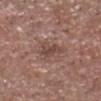workup = no biopsy performed (imaged during a skin exam)
diameter = ≈3.5 mm
automated lesion analysis = a border-irregularity rating of about 4.5/10, a within-lesion color-variation index near 2/10, and a peripheral color-asymmetry measure near 0.5; a detector confidence of about 100 out of 100 that the crop contains a lesion
image = ~15 mm crop, total-body skin-cancer survey
site = the head or neck
subject = male, aged 53–57
tile lighting = white-light